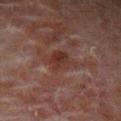Captured during whole-body skin photography for melanoma surveillance; the lesion was not biopsied. A 15 mm crop from a total-body photograph taken for skin-cancer surveillance. The subject is a male aged 68 to 72. Located on the left lower leg.